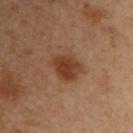• workup · imaged on a skin check; not biopsied
• location · the right upper arm
• image source · total-body-photography crop, ~15 mm field of view
• patient · male, aged 53 to 57